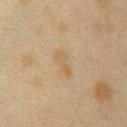Assessment: Recorded during total-body skin imaging; not selected for excision or biopsy. Clinical summary: The lesion is located on the left upper arm. A female patient, about 40 years old. A region of skin cropped from a whole-body photographic capture, roughly 15 mm wide. An algorithmic analysis of the crop reported a lesion color around L≈49 a*≈12 b*≈32 in CIELAB, roughly 5 lightness units darker than nearby skin, and a normalized lesion–skin contrast near 5.5. This is a cross-polarized tile.